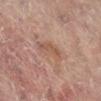The lesion is located on the left leg.
A close-up tile cropped from a whole-body skin photograph, about 15 mm across.
A female patient, aged 78–82.
Approximately 3.5 mm at its widest.
Captured under cross-polarized illumination.
An algorithmic analysis of the crop reported a mean CIELAB color near L≈48 a*≈18 b*≈26 and a lesion–skin lightness drop of about 6. It also reported border irregularity of about 3.5 on a 0–10 scale, a color-variation rating of about 3.5/10, and radial color variation of about 1.5.